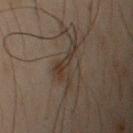{"biopsy_status": "not biopsied; imaged during a skin examination", "site": "right upper arm", "lesion_size": {"long_diameter_mm_approx": 5.5}, "patient": {"sex": "male", "age_approx": 50}, "image": {"source": "total-body photography crop", "field_of_view_mm": 15}, "lighting": "cross-polarized", "automated_metrics": {"area_mm2_approx": 8.0, "eccentricity": 0.85, "shape_asymmetry": 0.7, "cielab_L": 26, "cielab_a": 9, "cielab_b": 17, "vs_skin_contrast_norm": 6.5, "lesion_detection_confidence_0_100": 90}}